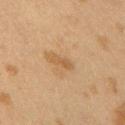workup: catalogued during a skin exam; not biopsied
illumination: cross-polarized illumination
image source: 15 mm crop, total-body photography
size: ≈2.5 mm
automated lesion analysis: a mean CIELAB color near L≈46 a*≈16 b*≈33 and a lesion–skin lightness drop of about 7; a border-irregularity rating of about 3.5/10, a color-variation rating of about 0/10, and a peripheral color-asymmetry measure near 0
patient: female, aged 38–42
anatomic site: the right upper arm